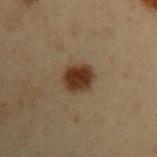No biopsy was performed on this lesion — it was imaged during a full skin examination and was not determined to be concerning. The subject is a male in their mid- to late 50s. Cropped from a whole-body photographic skin survey; the tile spans about 15 mm. The total-body-photography lesion software estimated an outline eccentricity of about 0.7 (0 = round, 1 = elongated). And it measured about 12 CIELAB-L* units darker than the surrounding skin. The analysis additionally found a border-irregularity index near 1.5/10 and internal color variation of about 2.5 on a 0–10 scale. The software also gave a classifier nevus-likeness of about 100/100 and a detector confidence of about 100 out of 100 that the crop contains a lesion. The recorded lesion diameter is about 3.5 mm. The lesion is located on the left upper arm. The tile uses cross-polarized illumination.